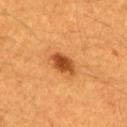No biopsy was performed on this lesion — it was imaged during a full skin examination and was not determined to be concerning. A 15 mm crop from a total-body photograph taken for skin-cancer surveillance. Located on the upper back. A male patient aged 58–62. This is a cross-polarized tile. About 4 mm across.